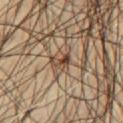  biopsy_status: not biopsied; imaged during a skin examination